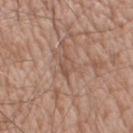Assessment: No biopsy was performed on this lesion — it was imaged during a full skin examination and was not determined to be concerning. Clinical summary: A male patient, about 60 years old. The tile uses white-light illumination. A region of skin cropped from a whole-body photographic capture, roughly 15 mm wide. From the right upper arm.